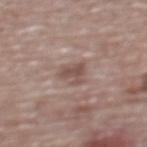Context: A female patient aged 63 to 67. From the upper back. This is a white-light tile. A 15 mm crop from a total-body photograph taken for skin-cancer surveillance. About 3.5 mm across.